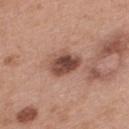workup = imaged on a skin check; not biopsied | image = ~15 mm tile from a whole-body skin photo | patient = female, approximately 40 years of age | diameter = ≈4 mm | illumination = white-light | site = the back.